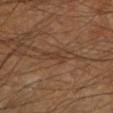Q: Was this lesion biopsied?
A: catalogued during a skin exam; not biopsied
Q: How was this image acquired?
A: ~15 mm crop, total-body skin-cancer survey
Q: Where on the body is the lesion?
A: the left lower leg
Q: What are the patient's age and sex?
A: male, about 60 years old